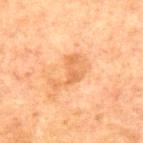This lesion was catalogued during total-body skin photography and was not selected for biopsy. Approximately 4.5 mm at its widest. On the upper back. The tile uses cross-polarized illumination. The lesion-visualizer software estimated a lesion–skin lightness drop of about 7 and a lesion-to-skin contrast of about 5.5 (normalized; higher = more distinct). The software also gave a lesion-detection confidence of about 100/100. The subject is a male in their 60s. A 15 mm crop from a total-body photograph taken for skin-cancer surveillance.